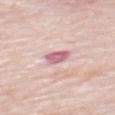Clinical impression:
This lesion was catalogued during total-body skin photography and was not selected for biopsy.
Background:
A male patient roughly 80 years of age. Captured under white-light illumination. Cropped from a whole-body photographic skin survey; the tile spans about 15 mm. Automated tile analysis of the lesion measured a lesion area of about 4.5 mm² and an eccentricity of roughly 0.7. The software also gave a lesion color around L≈63 a*≈27 b*≈17 in CIELAB, about 15 CIELAB-L* units darker than the surrounding skin, and a lesion-to-skin contrast of about 9 (normalized; higher = more distinct). And it measured a within-lesion color-variation index near 2/10 and a peripheral color-asymmetry measure near 1. From the mid back.